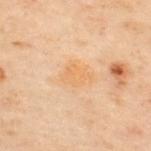Captured during whole-body skin photography for melanoma surveillance; the lesion was not biopsied. The tile uses cross-polarized illumination. The lesion's longest dimension is about 3 mm. The patient is a male aged 48 to 52. A lesion tile, about 15 mm wide, cut from a 3D total-body photograph. On the back.